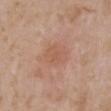Imaged during a routine full-body skin examination; the lesion was not biopsied and no histopathology is available. A roughly 15 mm field-of-view crop from a total-body skin photograph. A female patient aged 33–37. Measured at roughly 3.5 mm in maximum diameter. On the right upper arm.